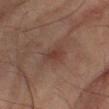Imaged during a routine full-body skin examination; the lesion was not biopsied and no histopathology is available.
This image is a 15 mm lesion crop taken from a total-body photograph.
The subject is a male approximately 75 years of age.
The lesion is on the leg.